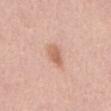notes: no biopsy performed (imaged during a skin exam)
lesion size: ~3 mm (longest diameter)
TBP lesion metrics: a footprint of about 4.5 mm² and an eccentricity of roughly 0.8; a mean CIELAB color near L≈63 a*≈23 b*≈31 and a lesion–skin lightness drop of about 10; an automated nevus-likeness rating near 90 out of 100
illumination: white-light illumination
subject: male, aged 53 to 57
image source: ~15 mm tile from a whole-body skin photo